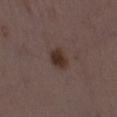Part of a total-body skin-imaging series; this lesion was reviewed on a skin check and was not flagged for biopsy. Captured under white-light illumination. An algorithmic analysis of the crop reported an outline eccentricity of about 0.7 (0 = round, 1 = elongated) and two-axis asymmetry of about 0.2. The software also gave roughly 9 lightness units darker than nearby skin. And it measured a classifier nevus-likeness of about 100/100 and a lesion-detection confidence of about 100/100. Approximately 3 mm at its widest. A 15 mm crop from a total-body photograph taken for skin-cancer surveillance. The lesion is on the right thigh. A female patient, roughly 35 years of age.